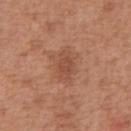Q: Was this lesion biopsied?
A: total-body-photography surveillance lesion; no biopsy
Q: What is the imaging modality?
A: ~15 mm crop, total-body skin-cancer survey
Q: What is the anatomic site?
A: the front of the torso
Q: How was the tile lit?
A: white-light illumination
Q: Who is the patient?
A: male, in their mid- to late 60s
Q: Lesion size?
A: ~3.5 mm (longest diameter)
Q: What did automated image analysis measure?
A: an area of roughly 6 mm² and a shape eccentricity near 0.75; a lesion color around L≈49 a*≈24 b*≈31 in CIELAB and a normalized lesion–skin contrast near 5.5; a nevus-likeness score of about 10/100 and lesion-presence confidence of about 100/100